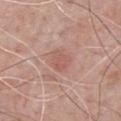The lesion was tiled from a total-body skin photograph and was not biopsied. Approximately 2.5 mm at its widest. From the front of the torso. Captured under white-light illumination. The lesion-visualizer software estimated an eccentricity of roughly 0.45 and a shape-asymmetry score of about 0.25 (0 = symmetric). It also reported a border-irregularity rating of about 2.5/10. The software also gave a nevus-likeness score of about 0/100. Cropped from a whole-body photographic skin survey; the tile spans about 15 mm. A male patient, in their 60s.